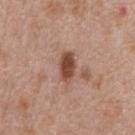Assessment:
Captured during whole-body skin photography for melanoma surveillance; the lesion was not biopsied.
Acquisition and patient details:
A male patient aged approximately 65. Longest diameter approximately 3.5 mm. A roughly 15 mm field-of-view crop from a total-body skin photograph. On the chest. The tile uses white-light illumination. Automated image analysis of the tile measured a nevus-likeness score of about 90/100 and a lesion-detection confidence of about 100/100.